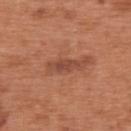notes: catalogued during a skin exam; not biopsied | TBP lesion metrics: two-axis asymmetry of about 0.4; an average lesion color of about L≈46 a*≈25 b*≈30 (CIELAB) and a normalized border contrast of about 7.5; internal color variation of about 0 on a 0–10 scale; a nevus-likeness score of about 0/100 and lesion-presence confidence of about 70/100 | body site: the upper back | lesion size: ~3 mm (longest diameter) | imaging modality: 15 mm crop, total-body photography | tile lighting: white-light illumination | patient: male, approximately 65 years of age.